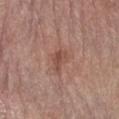Notes:
• follow-up · total-body-photography surveillance lesion; no biopsy
• subject · female, aged approximately 65
• acquisition · ~15 mm tile from a whole-body skin photo
• lesion diameter · ~3 mm (longest diameter)
• lighting · white-light illumination
• TBP lesion metrics · border irregularity of about 4 on a 0–10 scale, a color-variation rating of about 2.5/10, and peripheral color asymmetry of about 1; a nevus-likeness score of about 0/100 and a detector confidence of about 100 out of 100 that the crop contains a lesion
• body site · the right forearm